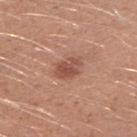notes: no biopsy performed (imaged during a skin exam)
location: the right upper arm
subject: male, aged approximately 25
acquisition: total-body-photography crop, ~15 mm field of view
diameter: ~3.5 mm (longest diameter)
image-analysis metrics: an area of roughly 6 mm² and a shape eccentricity near 0.75; a lesion color around L≈51 a*≈24 b*≈29 in CIELAB and a normalized border contrast of about 7; border irregularity of about 2.5 on a 0–10 scale, a color-variation rating of about 4/10, and peripheral color asymmetry of about 1